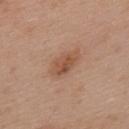- biopsy status · imaged on a skin check; not biopsied
- body site · the upper back
- size · ~4 mm (longest diameter)
- subject · male, about 55 years old
- image source · 15 mm crop, total-body photography